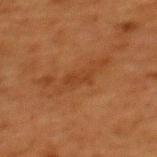This lesion was catalogued during total-body skin photography and was not selected for biopsy. Located on the mid back. About 3 mm across. A female subject approximately 50 years of age. An algorithmic analysis of the crop reported a footprint of about 3 mm², an outline eccentricity of about 0.9 (0 = round, 1 = elongated), and two-axis asymmetry of about 0.35. And it measured about 5 CIELAB-L* units darker than the surrounding skin and a normalized lesion–skin contrast near 5. It also reported a nevus-likeness score of about 0/100 and lesion-presence confidence of about 100/100. Captured under cross-polarized illumination. A 15 mm close-up extracted from a 3D total-body photography capture.